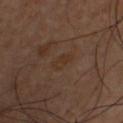biopsy status = catalogued during a skin exam; not biopsied | image = ~15 mm crop, total-body skin-cancer survey | diameter = ~3 mm (longest diameter) | subject = male, aged around 40 | site = the chest.